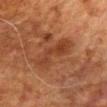Q: Is there a histopathology result?
A: no biopsy performed (imaged during a skin exam)